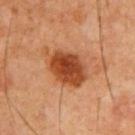– illumination · cross-polarized illumination
– patient · male, aged around 65
– location · the chest
– imaging modality · ~15 mm tile from a whole-body skin photo